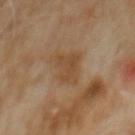workup = no biopsy performed (imaged during a skin exam)
location = the abdomen
image = total-body-photography crop, ~15 mm field of view
subject = male, in their 60s